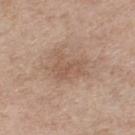Q: Was a biopsy performed?
A: imaged on a skin check; not biopsied
Q: How was this image acquired?
A: 15 mm crop, total-body photography
Q: Lesion size?
A: ≈4.5 mm
Q: Who is the patient?
A: female, approximately 55 years of age
Q: What did automated image analysis measure?
A: a lesion area of about 10 mm², a shape eccentricity near 0.65, and a symmetry-axis asymmetry near 0.45; a lesion–skin lightness drop of about 7 and a normalized border contrast of about 5
Q: Lesion location?
A: the right lower leg
Q: Illumination type?
A: white-light illumination